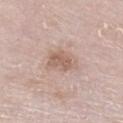Recorded during total-body skin imaging; not selected for excision or biopsy. Located on the right lower leg. A male subject approximately 80 years of age. About 4 mm across. This is a white-light tile. A roughly 15 mm field-of-view crop from a total-body skin photograph.